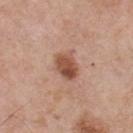follow-up: imaged on a skin check; not biopsied | illumination: white-light | body site: the upper back | patient: male, aged 53 to 57 | acquisition: total-body-photography crop, ~15 mm field of view | size: ~3.5 mm (longest diameter).